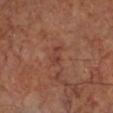{"biopsy_status": "not biopsied; imaged during a skin examination", "lesion_size": {"long_diameter_mm_approx": 3.0}, "site": "leg", "lighting": "cross-polarized", "automated_metrics": {"area_mm2_approx": 3.5, "eccentricity": 0.9, "cielab_L": 40, "cielab_a": 24, "cielab_b": 26, "vs_skin_darker_L": 6.0, "vs_skin_contrast_norm": 5.5, "border_irregularity_0_10": 3.0, "color_variation_0_10": 2.0, "nevus_likeness_0_100": 0, "lesion_detection_confidence_0_100": 100}, "patient": {"sex": "male", "age_approx": 65}, "image": {"source": "total-body photography crop", "field_of_view_mm": 15}}